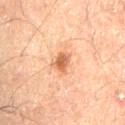  biopsy_status: not biopsied; imaged during a skin examination
  lighting: cross-polarized
  image:
    source: total-body photography crop
    field_of_view_mm: 15
  patient:
    sex: male
    age_approx: 60
  site: lower back
  lesion_size:
    long_diameter_mm_approx: 2.5